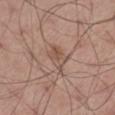Recorded during total-body skin imaging; not selected for excision or biopsy. Longest diameter approximately 3 mm. This image is a 15 mm lesion crop taken from a total-body photograph. On the leg. Captured under white-light illumination. Automated image analysis of the tile measured a footprint of about 6 mm², an eccentricity of roughly 0.7, and a symmetry-axis asymmetry near 0.2. And it measured a lesion-to-skin contrast of about 5.5 (normalized; higher = more distinct). The software also gave border irregularity of about 2 on a 0–10 scale, a color-variation rating of about 4/10, and radial color variation of about 1.5. The subject is a male aged approximately 55.